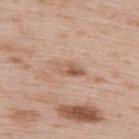The lesion was photographed on a routine skin check and not biopsied; there is no pathology result. The total-body-photography lesion software estimated a lesion area of about 5 mm². The software also gave border irregularity of about 3.5 on a 0–10 scale, internal color variation of about 7.5 on a 0–10 scale, and peripheral color asymmetry of about 3.5. The software also gave a nevus-likeness score of about 0/100 and lesion-presence confidence of about 100/100. Captured under white-light illumination. Cropped from a total-body skin-imaging series; the visible field is about 15 mm. A male patient, aged 48–52. The lesion's longest dimension is about 4 mm. Located on the upper back.